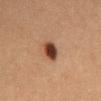Recorded during total-body skin imaging; not selected for excision or biopsy.
Captured under cross-polarized illumination.
From the front of the torso.
The total-body-photography lesion software estimated a border-irregularity index near 1.5/10 and a within-lesion color-variation index near 4.5/10. And it measured a nevus-likeness score of about 100/100 and a detector confidence of about 100 out of 100 that the crop contains a lesion.
A lesion tile, about 15 mm wide, cut from a 3D total-body photograph.
A female subject aged 58 to 62.